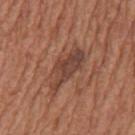Case summary:
– body site · the back
– TBP lesion metrics · border irregularity of about 5 on a 0–10 scale, internal color variation of about 3.5 on a 0–10 scale, and a peripheral color-asymmetry measure near 1
– patient · male, aged around 65
– image · 15 mm crop, total-body photography
– lesion diameter · ≈5.5 mm
– tile lighting · white-light illumination